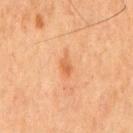notes: imaged on a skin check; not biopsied
patient: male, aged approximately 65
illumination: cross-polarized illumination
imaging modality: ~15 mm tile from a whole-body skin photo
body site: the chest